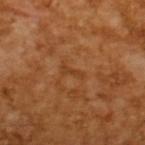workup — imaged on a skin check; not biopsied
imaging modality — ~15 mm crop, total-body skin-cancer survey
subject — male, aged around 65
size — ≈2.5 mm
image-analysis metrics — an area of roughly 2 mm², a shape eccentricity near 0.95, and a shape-asymmetry score of about 0.45 (0 = symmetric); an average lesion color of about L≈39 a*≈24 b*≈37 (CIELAB), about 6 CIELAB-L* units darker than the surrounding skin, and a lesion-to-skin contrast of about 5.5 (normalized; higher = more distinct)
lighting — cross-polarized